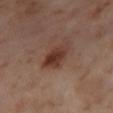No biopsy was performed on this lesion — it was imaged during a full skin examination and was not determined to be concerning.